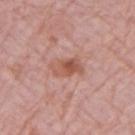lesion size=≈4 mm
site=the left upper arm
tile lighting=white-light illumination
image source=~15 mm crop, total-body skin-cancer survey
subject=female, aged approximately 65